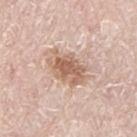Part of a total-body skin-imaging series; this lesion was reviewed on a skin check and was not flagged for biopsy.
About 5.5 mm across.
The tile uses white-light illumination.
A male subject about 80 years old.
Cropped from a total-body skin-imaging series; the visible field is about 15 mm.
Automated image analysis of the tile measured a lesion area of about 13 mm², an outline eccentricity of about 0.8 (0 = round, 1 = elongated), and a shape-asymmetry score of about 0.2 (0 = symmetric). It also reported a detector confidence of about 100 out of 100 that the crop contains a lesion.
The lesion is on the left thigh.